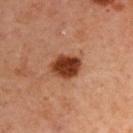• follow-up: total-body-photography surveillance lesion; no biopsy
• lesion size: about 3.5 mm
• subject: male, aged approximately 60
• acquisition: total-body-photography crop, ~15 mm field of view
• body site: the left upper arm
• TBP lesion metrics: an area of roughly 8 mm² and a shape-asymmetry score of about 0.15 (0 = symmetric); a color-variation rating of about 3/10 and peripheral color asymmetry of about 1
• tile lighting: cross-polarized